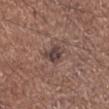The lesion is located on the leg.
The patient is a female aged around 50.
A 15 mm close-up tile from a total-body photography series done for melanoma screening.
The lesion's longest dimension is about 3 mm.